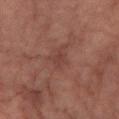<tbp_lesion>
  <image>
    <source>total-body photography crop</source>
    <field_of_view_mm>15</field_of_view_mm>
  </image>
  <patient>
    <sex>female</sex>
    <age_approx>60</age_approx>
  </patient>
  <site>leg</site>
</tbp_lesion>